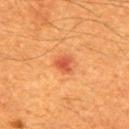This lesion was catalogued during total-body skin photography and was not selected for biopsy. A close-up tile cropped from a whole-body skin photograph, about 15 mm across. A male subject, aged 58 to 62. An algorithmic analysis of the crop reported a detector confidence of about 100 out of 100 that the crop contains a lesion. The lesion is located on the upper back.